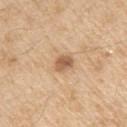Clinical impression: The lesion was tiled from a total-body skin photograph and was not biopsied. Image and clinical context: Imaged with white-light lighting. A 15 mm crop from a total-body photograph taken for skin-cancer surveillance. On the left upper arm. A male patient, aged approximately 70.